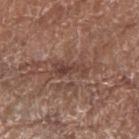image: ~15 mm tile from a whole-body skin photo; anatomic site: the arm; subject: female, aged around 75; lighting: white-light illumination; TBP lesion metrics: a footprint of about 5 mm² and an outline eccentricity of about 0.95 (0 = round, 1 = elongated); diameter: about 4 mm.